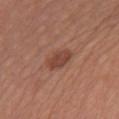<case>
  <biopsy_status>not biopsied; imaged during a skin examination</biopsy_status>
  <patient>
    <sex>female</sex>
    <age_approx>55</age_approx>
  </patient>
  <lesion_size>
    <long_diameter_mm_approx>3.5</long_diameter_mm_approx>
  </lesion_size>
  <image>
    <source>total-body photography crop</source>
    <field_of_view_mm>15</field_of_view_mm>
  </image>
  <site>chest</site>
  <lighting>white-light</lighting>
</case>